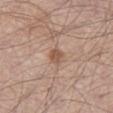workup: total-body-photography surveillance lesion; no biopsy
size: ≈2.5 mm
automated metrics: a lesion color around L≈54 a*≈19 b*≈29 in CIELAB and a lesion-to-skin contrast of about 7 (normalized; higher = more distinct); a border-irregularity rating of about 2/10, a color-variation rating of about 1.5/10, and radial color variation of about 0.5; a classifier nevus-likeness of about 45/100
site: the left thigh
image: ~15 mm tile from a whole-body skin photo
patient: male, aged 53 to 57
lighting: white-light illumination